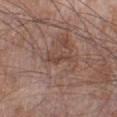Notes:
- notes: total-body-photography surveillance lesion; no biopsy
- subject: male, approximately 60 years of age
- anatomic site: the left lower leg
- image-analysis metrics: an average lesion color of about L≈44 a*≈18 b*≈24 (CIELAB), about 7 CIELAB-L* units darker than the surrounding skin, and a lesion-to-skin contrast of about 6 (normalized; higher = more distinct); a classifier nevus-likeness of about 0/100 and lesion-presence confidence of about 50/100
- lesion diameter: about 4 mm
- tile lighting: white-light
- imaging modality: 15 mm crop, total-body photography